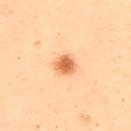The lesion was tiled from a total-body skin photograph and was not biopsied. An algorithmic analysis of the crop reported a border-irregularity rating of about 1.5/10, internal color variation of about 3.5 on a 0–10 scale, and peripheral color asymmetry of about 1. It also reported a nevus-likeness score of about 100/100. A 15 mm close-up tile from a total-body photography series done for melanoma screening. From the upper back. Approximately 3 mm at its widest. Captured under cross-polarized illumination. A female subject, roughly 50 years of age.